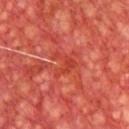Assessment:
Imaged during a routine full-body skin examination; the lesion was not biopsied and no histopathology is available.